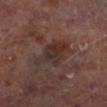Clinical impression:
The lesion was photographed on a routine skin check and not biopsied; there is no pathology result.
Clinical summary:
A lesion tile, about 15 mm wide, cut from a 3D total-body photograph. About 5.5 mm across. This is a cross-polarized tile. From the left lower leg. A male subject about 65 years old.